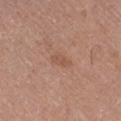biopsy status=total-body-photography surveillance lesion; no biopsy
illumination=white-light illumination
site=the right lower leg
subject=female, roughly 50 years of age
imaging modality=~15 mm tile from a whole-body skin photo
lesion size=~3 mm (longest diameter)